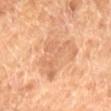patient:
  sex: female
  age_approx: 70
automated_metrics:
  area_mm2_approx: 14.0
  eccentricity: 0.4
  shape_asymmetry: 0.65
  cielab_L: 64
  cielab_a: 22
  cielab_b: 36
  vs_skin_contrast_norm: 5.0
  color_variation_0_10: 2.5
  peripheral_color_asymmetry: 0.5
lesion_size:
  long_diameter_mm_approx: 5.0
image:
  source: total-body photography crop
  field_of_view_mm: 15
site: leg
lighting: cross-polarized